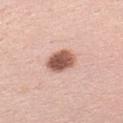Imaged during a routine full-body skin examination; the lesion was not biopsied and no histopathology is available.
The recorded lesion diameter is about 3.5 mm.
The patient is a female approximately 35 years of age.
The tile uses white-light illumination.
On the left upper arm.
A 15 mm close-up tile from a total-body photography series done for melanoma screening.